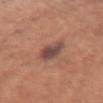Captured during whole-body skin photography for melanoma surveillance; the lesion was not biopsied. Automated image analysis of the tile measured an area of roughly 7.5 mm² and a shape eccentricity near 0.65. The analysis additionally found a normalized border contrast of about 9.5. It also reported border irregularity of about 2 on a 0–10 scale and radial color variation of about 1.5. About 3.5 mm across. From the right upper arm. The patient is a female in their 50s. Captured under white-light illumination. A 15 mm close-up extracted from a 3D total-body photography capture.